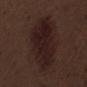<lesion>
<image>
  <source>total-body photography crop</source>
  <field_of_view_mm>15</field_of_view_mm>
</image>
<patient>
  <sex>male</sex>
  <age_approx>70</age_approx>
</patient>
<site>right thigh</site>
<automated_metrics>
  <cielab_L>19</cielab_L>
  <cielab_a>16</cielab_a>
  <cielab_b>16</cielab_b>
  <vs_skin_darker_L>7.0</vs_skin_darker_L>
  <nevus_likeness_0_100>80</nevus_likeness_0_100>
  <lesion_detection_confidence_0_100>100</lesion_detection_confidence_0_100>
</automated_metrics>
<lighting>white-light</lighting>
<lesion_size>
  <long_diameter_mm_approx>9.5</long_diameter_mm_approx>
</lesion_size>
</lesion>